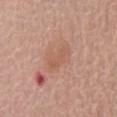Part of a total-body skin-imaging series; this lesion was reviewed on a skin check and was not flagged for biopsy.
A male subject approximately 80 years of age.
This image is a 15 mm lesion crop taken from a total-body photograph.
Approximately 4 mm at its widest.
Located on the chest.
Captured under white-light illumination.